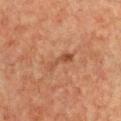Clinical impression: The lesion was tiled from a total-body skin photograph and was not biopsied. Acquisition and patient details: A 15 mm close-up extracted from a 3D total-body photography capture. A female subject aged approximately 55. The lesion is on the front of the torso. This is a cross-polarized tile.